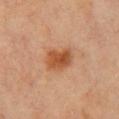Assessment: The lesion was photographed on a routine skin check and not biopsied; there is no pathology result. Context: This is a cross-polarized tile. The subject is a female aged approximately 65. This image is a 15 mm lesion crop taken from a total-body photograph. The lesion is on the chest.